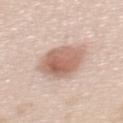Case summary:
– lesion diameter — about 5 mm
– site — the upper back
– image — ~15 mm tile from a whole-body skin photo
– tile lighting — white-light
– subject — female, roughly 40 years of age